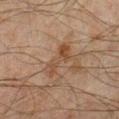Clinical impression: Part of a total-body skin-imaging series; this lesion was reviewed on a skin check and was not flagged for biopsy. Clinical summary: On the left lower leg. The tile uses cross-polarized illumination. Cropped from a total-body skin-imaging series; the visible field is about 15 mm. The lesion's longest dimension is about 4 mm. The patient is a male about 45 years old.